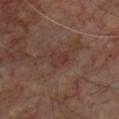follow-up — catalogued during a skin exam; not biopsied
body site — the chest
subject — male, in their 70s
acquisition — total-body-photography crop, ~15 mm field of view
lighting — cross-polarized illumination